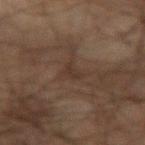notes: no biopsy performed (imaged during a skin exam); lesion diameter: ≈2.5 mm; patient: male, about 70 years old; tile lighting: cross-polarized; imaging modality: ~15 mm tile from a whole-body skin photo; anatomic site: the arm.